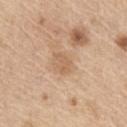| field | value |
|---|---|
| notes | no biopsy performed (imaged during a skin exam) |
| size | ~2.5 mm (longest diameter) |
| body site | the abdomen |
| acquisition | total-body-photography crop, ~15 mm field of view |
| TBP lesion metrics | a footprint of about 3.5 mm², an eccentricity of roughly 0.75, and a symmetry-axis asymmetry near 0.45; a classifier nevus-likeness of about 0/100 and a detector confidence of about 100 out of 100 that the crop contains a lesion |
| subject | male, about 70 years old |